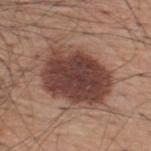biopsy status: no biopsy performed (imaged during a skin exam)
illumination: white-light
image-analysis metrics: a lesion area of about 35 mm², a shape eccentricity near 0.7, and a shape-asymmetry score of about 0.2 (0 = symmetric); a mean CIELAB color near L≈41 a*≈20 b*≈24, about 15 CIELAB-L* units darker than the surrounding skin, and a normalized lesion–skin contrast near 11.5; internal color variation of about 5 on a 0–10 scale and a peripheral color-asymmetry measure near 1.5; an automated nevus-likeness rating near 50 out of 100 and a detector confidence of about 100 out of 100 that the crop contains a lesion
location: the upper back
imaging modality: 15 mm crop, total-body photography
subject: male, aged around 60